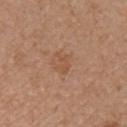Notes:
– follow-up: catalogued during a skin exam; not biopsied
– patient: female, aged around 35
– image-analysis metrics: an average lesion color of about L≈51 a*≈20 b*≈33 (CIELAB) and a normalized lesion–skin contrast near 5.5; a classifier nevus-likeness of about 0/100 and a detector confidence of about 100 out of 100 that the crop contains a lesion
– site: the chest
– tile lighting: white-light
– lesion size: ≈2.5 mm
– imaging modality: ~15 mm tile from a whole-body skin photo